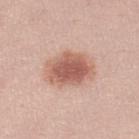The lesion was photographed on a routine skin check and not biopsied; there is no pathology result.
Longest diameter approximately 5.5 mm.
From the left lower leg.
A female subject, about 25 years old.
A roughly 15 mm field-of-view crop from a total-body skin photograph.